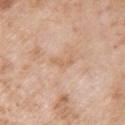From the arm.
A female subject aged 68 to 72.
A close-up tile cropped from a whole-body skin photograph, about 15 mm across.